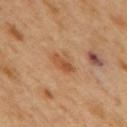| field | value |
|---|---|
| notes | total-body-photography surveillance lesion; no biopsy |
| patient | male, approximately 50 years of age |
| imaging modality | ~15 mm tile from a whole-body skin photo |
| site | the back |
| lesion size | ~3.5 mm (longest diameter) |
| illumination | cross-polarized |
| automated lesion analysis | a mean CIELAB color near L≈52 a*≈23 b*≈37, roughly 9 lightness units darker than nearby skin, and a normalized border contrast of about 6.5; border irregularity of about 3 on a 0–10 scale, internal color variation of about 4.5 on a 0–10 scale, and peripheral color asymmetry of about 1.5; a classifier nevus-likeness of about 60/100 and a lesion-detection confidence of about 100/100 |